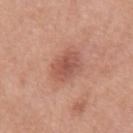Impression: Captured during whole-body skin photography for melanoma surveillance; the lesion was not biopsied. Acquisition and patient details: Captured under white-light illumination. A male subject, aged 68 to 72. Measured at roughly 3.5 mm in maximum diameter. A roughly 15 mm field-of-view crop from a total-body skin photograph. Located on the mid back. Automated tile analysis of the lesion measured an area of roughly 7.5 mm² and a shape-asymmetry score of about 0.15 (0 = symmetric). The analysis additionally found a classifier nevus-likeness of about 55/100 and lesion-presence confidence of about 100/100.